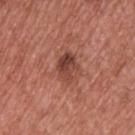- patient: male, aged approximately 55
- imaging modality: 15 mm crop, total-body photography
- site: the upper back
- lesion diameter: about 3.5 mm
- illumination: white-light